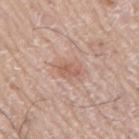Recorded during total-body skin imaging; not selected for excision or biopsy.
A 15 mm close-up extracted from a 3D total-body photography capture.
The total-body-photography lesion software estimated a lesion color around L≈60 a*≈20 b*≈28 in CIELAB, about 8 CIELAB-L* units darker than the surrounding skin, and a normalized border contrast of about 5.5.
The patient is a male in their mid-70s.
The lesion is on the arm.